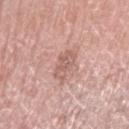| field | value |
|---|---|
| notes | catalogued during a skin exam; not biopsied |
| lighting | white-light illumination |
| patient | female, aged around 60 |
| acquisition | ~15 mm tile from a whole-body skin photo |
| automated lesion analysis | two-axis asymmetry of about 0.35; a lesion-to-skin contrast of about 6 (normalized; higher = more distinct); a border-irregularity rating of about 4.5/10, a within-lesion color-variation index near 2.5/10, and radial color variation of about 1 |
| anatomic site | the right upper arm |